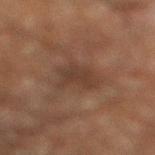Notes:
- workup: no biopsy performed (imaged during a skin exam)
- diameter: ~3.5 mm (longest diameter)
- body site: the leg
- image source: ~15 mm tile from a whole-body skin photo
- patient: male, aged 63 to 67